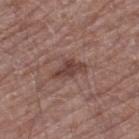biopsy status=catalogued during a skin exam; not biopsied
patient=male, aged approximately 70
anatomic site=the left thigh
image source=total-body-photography crop, ~15 mm field of view